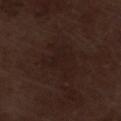Assessment:
This lesion was catalogued during total-body skin photography and was not selected for biopsy.
Acquisition and patient details:
The lesion is located on the leg. A male subject, aged 68–72. A lesion tile, about 15 mm wide, cut from a 3D total-body photograph. About 5 mm across.